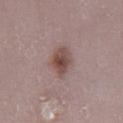Part of a total-body skin-imaging series; this lesion was reviewed on a skin check and was not flagged for biopsy. A close-up tile cropped from a whole-body skin photograph, about 15 mm across. Located on the leg. The total-body-photography lesion software estimated an area of roughly 8 mm², an eccentricity of roughly 0.7, and a shape-asymmetry score of about 0.25 (0 = symmetric). The software also gave a border-irregularity rating of about 2.5/10, internal color variation of about 5 on a 0–10 scale, and a peripheral color-asymmetry measure near 1.5. Longest diameter approximately 4 mm. A male patient about 80 years old.